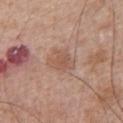The lesion was photographed on a routine skin check and not biopsied; there is no pathology result. Longest diameter approximately 3.5 mm. A 15 mm close-up tile from a total-body photography series done for melanoma screening. The subject is a male aged 63 to 67. Automated tile analysis of the lesion measured a lesion color around L≈55 a*≈20 b*≈28 in CIELAB, about 7 CIELAB-L* units darker than the surrounding skin, and a normalized lesion–skin contrast near 5. It also reported border irregularity of about 2 on a 0–10 scale, internal color variation of about 2.5 on a 0–10 scale, and a peripheral color-asymmetry measure near 1. Imaged with white-light lighting. The lesion is located on the left upper arm.